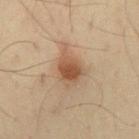workup — no biopsy performed (imaged during a skin exam) | subject — male, aged 63–67 | site — the back | tile lighting — cross-polarized | image source — ~15 mm crop, total-body skin-cancer survey | lesion diameter — about 5 mm.